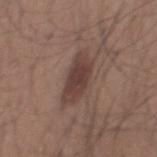follow-up: no biopsy performed (imaged during a skin exam) | diameter: ~5 mm (longest diameter) | acquisition: ~15 mm crop, total-body skin-cancer survey | subject: male, aged 33 to 37 | lighting: white-light | automated lesion analysis: a footprint of about 11 mm², an outline eccentricity of about 0.9 (0 = round, 1 = elongated), and a symmetry-axis asymmetry near 0.2; an automated nevus-likeness rating near 90 out of 100 | body site: the mid back.